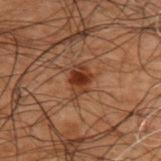follow-up — total-body-photography surveillance lesion; no biopsy | lesion size — ≈3.5 mm | anatomic site — the upper back | acquisition — total-body-photography crop, ~15 mm field of view | patient — male, aged around 50.